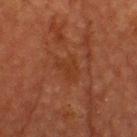* workup · no biopsy performed (imaged during a skin exam)
* lesion size · ≈3 mm
* lighting · cross-polarized
* subject · male, roughly 60 years of age
* body site · the head or neck
* image-analysis metrics · a border-irregularity rating of about 4.5/10, a within-lesion color-variation index near 1/10, and peripheral color asymmetry of about 0.5
* imaging modality · ~15 mm crop, total-body skin-cancer survey